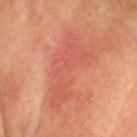About 9 mm across. Imaged with cross-polarized lighting. Cropped from a total-body skin-imaging series; the visible field is about 15 mm. A male subject roughly 55 years of age. The lesion is on the head or neck.